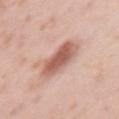The recorded lesion diameter is about 4.5 mm. The lesion is on the left upper arm. A region of skin cropped from a whole-body photographic capture, roughly 15 mm wide. A female patient aged approximately 40.